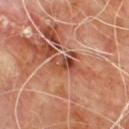{"site": "upper back", "patient": {"sex": "male", "age_approx": 60}, "image": {"source": "total-body photography crop", "field_of_view_mm": 15}}